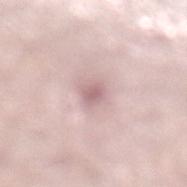No biopsy was performed on this lesion — it was imaged during a full skin examination and was not determined to be concerning.
Located on the left lower leg.
The patient is a male approximately 50 years of age.
Cropped from a total-body skin-imaging series; the visible field is about 15 mm.
The lesion's longest dimension is about 2.5 mm.
Captured under white-light illumination.